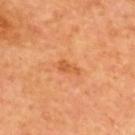Recorded during total-body skin imaging; not selected for excision or biopsy.
This image is a 15 mm lesion crop taken from a total-body photograph.
On the upper back.
The subject is a male approximately 65 years of age.